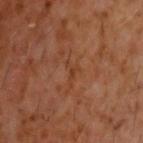Recorded during total-body skin imaging; not selected for excision or biopsy. The patient is a male about 60 years old. Captured under cross-polarized illumination. Cropped from a whole-body photographic skin survey; the tile spans about 15 mm. Approximately 3 mm at its widest. The total-body-photography lesion software estimated a classifier nevus-likeness of about 0/100 and a lesion-detection confidence of about 95/100. On the head or neck.